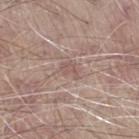Imaged during a routine full-body skin examination; the lesion was not biopsied and no histopathology is available. About 2.5 mm across. A roughly 15 mm field-of-view crop from a total-body skin photograph. From the right thigh. Captured under white-light illumination. A male subject in their mid- to late 60s.